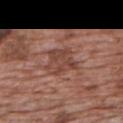follow-up — catalogued during a skin exam; not biopsied | subject — male, aged 68 to 72 | image source — ~15 mm crop, total-body skin-cancer survey | anatomic site — the back | image-analysis metrics — a lesion color around L≈44 a*≈22 b*≈26 in CIELAB, roughly 8 lightness units darker than nearby skin, and a lesion-to-skin contrast of about 6.5 (normalized; higher = more distinct).